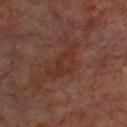Clinical impression:
Recorded during total-body skin imaging; not selected for excision or biopsy.
Acquisition and patient details:
Measured at roughly 5.5 mm in maximum diameter. The subject is a male aged 68 to 72. The lesion-visualizer software estimated a border-irregularity rating of about 5.5/10, internal color variation of about 3 on a 0–10 scale, and peripheral color asymmetry of about 1. It also reported an automated nevus-likeness rating near 0 out of 100 and lesion-presence confidence of about 100/100. The lesion is located on the chest. A 15 mm close-up extracted from a 3D total-body photography capture. Imaged with cross-polarized lighting.